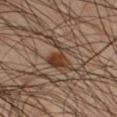Clinical impression:
Imaged during a routine full-body skin examination; the lesion was not biopsied and no histopathology is available.
Image and clinical context:
A male patient, in their mid-40s. Longest diameter approximately 4 mm. A 15 mm close-up tile from a total-body photography series done for melanoma screening. The lesion-visualizer software estimated an area of roughly 7 mm², a shape eccentricity near 0.8, and a symmetry-axis asymmetry near 0.6. The software also gave a lesion color around L≈36 a*≈17 b*≈27 in CIELAB and a normalized lesion–skin contrast near 9. And it measured an automated nevus-likeness rating near 60 out of 100 and a lesion-detection confidence of about 95/100. The tile uses cross-polarized illumination. On the right thigh.